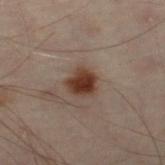Imaged during a routine full-body skin examination; the lesion was not biopsied and no histopathology is available.